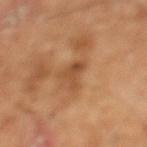  biopsy_status: not biopsied; imaged during a skin examination
  lesion_size:
    long_diameter_mm_approx: 2.5
  image:
    source: total-body photography crop
    field_of_view_mm: 15
  automated_metrics:
    area_mm2_approx: 4.0
    eccentricity: 0.05
    shape_asymmetry: 0.5
    border_irregularity_0_10: 5.0
    color_variation_0_10: 3.0
    peripheral_color_asymmetry: 1.0
  site: left lower leg
  patient:
    sex: male
    age_approx: 65